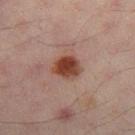The lesion was tiled from a total-body skin photograph and was not biopsied. The subject is a male roughly 45 years of age. Imaged with cross-polarized lighting. Automated image analysis of the tile measured a footprint of about 7 mm² and a symmetry-axis asymmetry near 0.2. And it measured border irregularity of about 1.5 on a 0–10 scale, a color-variation rating of about 4.5/10, and a peripheral color-asymmetry measure near 1.5. On the right thigh. Cropped from a whole-body photographic skin survey; the tile spans about 15 mm.